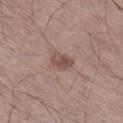– image · total-body-photography crop, ~15 mm field of view
– body site · the right lower leg
– subject · male, aged 53 to 57